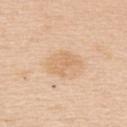| feature | finding |
|---|---|
| patient | female, aged approximately 45 |
| body site | the upper back |
| image | ~15 mm tile from a whole-body skin photo |